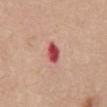Imaged during a routine full-body skin examination; the lesion was not biopsied and no histopathology is available.
A close-up tile cropped from a whole-body skin photograph, about 15 mm across.
A female patient aged around 65.
The lesion is on the abdomen.